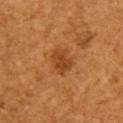Clinical impression: This lesion was catalogued during total-body skin photography and was not selected for biopsy. Context: Cropped from a total-body skin-imaging series; the visible field is about 15 mm. A female subject, aged approximately 55. Located on the arm.